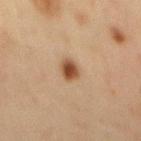follow-up: no biopsy performed (imaged during a skin exam); lesion diameter: ~3 mm (longest diameter); image: ~15 mm crop, total-body skin-cancer survey; illumination: cross-polarized illumination; subject: male, aged 43 to 47; body site: the mid back.